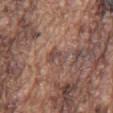biopsy status: total-body-photography surveillance lesion; no biopsy
image: 15 mm crop, total-body photography
subject: male, in their mid-70s
tile lighting: white-light
size: ~3 mm (longest diameter)
body site: the chest